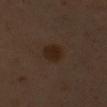notes: catalogued during a skin exam; not biopsied
illumination: cross-polarized
subject: male, about 50 years old
diameter: ~3.5 mm (longest diameter)
TBP lesion metrics: an area of roughly 6.5 mm², an eccentricity of roughly 0.7, and two-axis asymmetry of about 0.15; a mean CIELAB color near L≈22 a*≈14 b*≈22, about 6 CIELAB-L* units darker than the surrounding skin, and a lesion-to-skin contrast of about 8.5 (normalized; higher = more distinct)
imaging modality: ~15 mm tile from a whole-body skin photo
site: the left upper arm